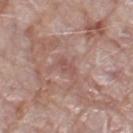Part of a total-body skin-imaging series; this lesion was reviewed on a skin check and was not flagged for biopsy. A 15 mm crop from a total-body photograph taken for skin-cancer surveillance. The recorded lesion diameter is about 2.5 mm. A female patient about 70 years old. Automated tile analysis of the lesion measured a border-irregularity rating of about 4.5/10 and a color-variation rating of about 0/10. It also reported an automated nevus-likeness rating near 0 out of 100 and a detector confidence of about 95 out of 100 that the crop contains a lesion. This is a white-light tile. On the left thigh.